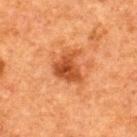The patient is a male aged approximately 50. From the upper back. Longest diameter approximately 3.5 mm. A 15 mm close-up tile from a total-body photography series done for melanoma screening.